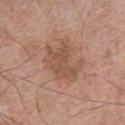biopsy status: imaged on a skin check; not biopsied
automated lesion analysis: a lesion-detection confidence of about 100/100
subject: male, approximately 65 years of age
site: the upper back
acquisition: total-body-photography crop, ~15 mm field of view
diameter: about 5.5 mm
illumination: white-light